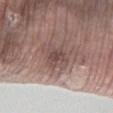Automated image analysis of the tile measured a classifier nevus-likeness of about 0/100 and lesion-presence confidence of about 100/100. The lesion is on the left lower leg. Captured under white-light illumination. The subject is a female aged 83 or older. Cropped from a whole-body photographic skin survey; the tile spans about 15 mm.